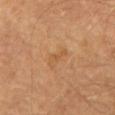Context: On the chest. The lesion's longest dimension is about 3.5 mm. A male patient, aged around 65. A roughly 15 mm field-of-view crop from a total-body skin photograph. Automated image analysis of the tile measured an area of roughly 3 mm² and an outline eccentricity of about 0.95 (0 = round, 1 = elongated). And it measured a mean CIELAB color near L≈48 a*≈19 b*≈35, a lesion–skin lightness drop of about 5, and a normalized border contrast of about 4.5. It also reported a border-irregularity rating of about 5.5/10.